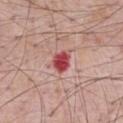Recorded during total-body skin imaging; not selected for excision or biopsy. The lesion is located on the front of the torso. A male subject, about 70 years old. A region of skin cropped from a whole-body photographic capture, roughly 15 mm wide.